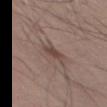Captured during whole-body skin photography for melanoma surveillance; the lesion was not biopsied.